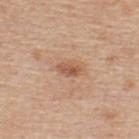Recorded during total-body skin imaging; not selected for excision or biopsy. An algorithmic analysis of the crop reported a lesion area of about 3.5 mm², an outline eccentricity of about 0.85 (0 = round, 1 = elongated), and a symmetry-axis asymmetry near 0.25. The analysis additionally found an average lesion color of about L≈56 a*≈22 b*≈33 (CIELAB), about 10 CIELAB-L* units darker than the surrounding skin, and a normalized border contrast of about 7. The analysis additionally found a detector confidence of about 100 out of 100 that the crop contains a lesion. The patient is a male about 60 years old. A region of skin cropped from a whole-body photographic capture, roughly 15 mm wide. The lesion is on the back.